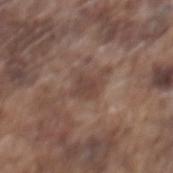This lesion was catalogued during total-body skin photography and was not selected for biopsy.
A male subject, in their mid- to late 70s.
A 15 mm close-up extracted from a 3D total-body photography capture.
On the mid back.